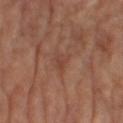{"biopsy_status": "not biopsied; imaged during a skin examination", "automated_metrics": {"eccentricity": 0.75, "shape_asymmetry": 0.3, "vs_skin_darker_L": 6.0, "vs_skin_contrast_norm": 5.0, "border_irregularity_0_10": 3.0, "color_variation_0_10": 2.5, "peripheral_color_asymmetry": 1.0, "nevus_likeness_0_100": 0}, "site": "right leg", "image": {"source": "total-body photography crop", "field_of_view_mm": 15}, "lesion_size": {"long_diameter_mm_approx": 2.5}, "patient": {"sex": "female", "age_approx": 65}, "lighting": "cross-polarized"}